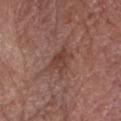Q: Was this lesion biopsied?
A: catalogued during a skin exam; not biopsied
Q: How was the tile lit?
A: white-light
Q: Automated lesion metrics?
A: an area of roughly 6 mm²; a lesion color around L≈41 a*≈21 b*≈24 in CIELAB, a lesion–skin lightness drop of about 8, and a normalized border contrast of about 6.5; a border-irregularity rating of about 3.5/10, a color-variation rating of about 2.5/10, and radial color variation of about 1; an automated nevus-likeness rating near 0 out of 100 and a detector confidence of about 100 out of 100 that the crop contains a lesion
Q: How large is the lesion?
A: ≈3 mm
Q: What is the anatomic site?
A: the head or neck
Q: How was this image acquired?
A: 15 mm crop, total-body photography
Q: Who is the patient?
A: male, approximately 75 years of age